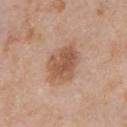<tbp_lesion>
<biopsy_status>not biopsied; imaged during a skin examination</biopsy_status>
<lighting>white-light</lighting>
<image>
  <source>total-body photography crop</source>
  <field_of_view_mm>15</field_of_view_mm>
</image>
<lesion_size>
  <long_diameter_mm_approx>5.0</long_diameter_mm_approx>
</lesion_size>
<patient>
  <sex>male</sex>
  <age_approx>60</age_approx>
</patient>
<site>chest</site>
</tbp_lesion>